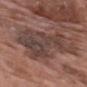Clinical impression: Recorded during total-body skin imaging; not selected for excision or biopsy. Image and clinical context: Imaged with white-light lighting. About 10.5 mm across. A 15 mm crop from a total-body photograph taken for skin-cancer surveillance. The total-body-photography lesion software estimated a lesion area of about 32 mm² and a shape eccentricity near 0.9. And it measured a border-irregularity index near 6.5/10, a color-variation rating of about 4.5/10, and peripheral color asymmetry of about 1.5. The lesion is on the upper back. A male patient, in their 70s.